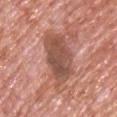Assessment:
This lesion was catalogued during total-body skin photography and was not selected for biopsy.
Acquisition and patient details:
About 6 mm across. The patient is a male roughly 75 years of age. Located on the front of the torso. This is a white-light tile. Cropped from a total-body skin-imaging series; the visible field is about 15 mm.